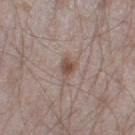<tbp_lesion>
<biopsy_status>not biopsied; imaged during a skin examination</biopsy_status>
<lighting>white-light</lighting>
<site>leg</site>
<lesion_size>
  <long_diameter_mm_approx>2.5</long_diameter_mm_approx>
</lesion_size>
<patient>
  <sex>male</sex>
  <age_approx>45</age_approx>
</patient>
<automated_metrics>
  <border_irregularity_0_10>3.0</border_irregularity_0_10>
  <color_variation_0_10>2.5</color_variation_0_10>
  <peripheral_color_asymmetry>0.5</peripheral_color_asymmetry>
</automated_metrics>
<image>
  <source>total-body photography crop</source>
  <field_of_view_mm>15</field_of_view_mm>
</image>
</tbp_lesion>